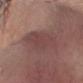biopsy status: total-body-photography surveillance lesion; no biopsy
location: the head or neck
subject: male, about 65 years old
image source: total-body-photography crop, ~15 mm field of view